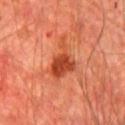No biopsy was performed on this lesion — it was imaged during a full skin examination and was not determined to be concerning. Captured under cross-polarized illumination. From the chest. A male patient, in their mid- to late 60s. An algorithmic analysis of the crop reported a mean CIELAB color near L≈44 a*≈33 b*≈37, a lesion–skin lightness drop of about 11, and a normalized border contrast of about 8.5. It also reported border irregularity of about 5.5 on a 0–10 scale and peripheral color asymmetry of about 1.5. A 15 mm close-up extracted from a 3D total-body photography capture. Approximately 5 mm at its widest.